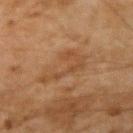notes — no biopsy performed (imaged during a skin exam) | diameter — ~5 mm (longest diameter) | lighting — cross-polarized illumination | subject — male, roughly 45 years of age | acquisition — 15 mm crop, total-body photography | site — the right upper arm.